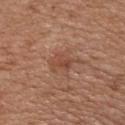workup: catalogued during a skin exam; not biopsied | imaging modality: total-body-photography crop, ~15 mm field of view | body site: the chest | automated lesion analysis: a footprint of about 3.5 mm² and a shape-asymmetry score of about 0.4 (0 = symmetric); border irregularity of about 4 on a 0–10 scale and radial color variation of about 1 | size: ~2.5 mm (longest diameter) | patient: male, in their mid- to late 50s.